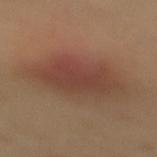<record>
  <biopsy_status>not biopsied; imaged during a skin examination</biopsy_status>
  <lighting>cross-polarized</lighting>
  <lesion_size>
    <long_diameter_mm_approx>8.0</long_diameter_mm_approx>
  </lesion_size>
  <patient>
    <sex>female</sex>
    <age_approx>50</age_approx>
  </patient>
  <site>mid back</site>
  <image>
    <source>total-body photography crop</source>
    <field_of_view_mm>15</field_of_view_mm>
  </image>
</record>